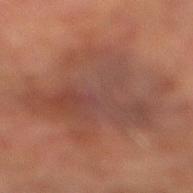Captured during whole-body skin photography for melanoma surveillance; the lesion was not biopsied.
A male subject, aged 73 to 77.
From the right lower leg.
A 15 mm close-up extracted from a 3D total-body photography capture.